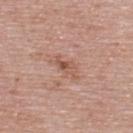<lesion>
  <biopsy_status>not biopsied; imaged during a skin examination</biopsy_status>
  <image>
    <source>total-body photography crop</source>
    <field_of_view_mm>15</field_of_view_mm>
  </image>
  <automated_metrics>
    <area_mm2_approx>4.5</area_mm2_approx>
    <shape_asymmetry>0.4</shape_asymmetry>
  </automated_metrics>
  <patient>
    <sex>female</sex>
    <age_approx>50</age_approx>
  </patient>
  <site>upper back</site>
  <lighting>white-light</lighting>
  <lesion_size>
    <long_diameter_mm_approx>3.0</long_diameter_mm_approx>
  </lesion_size>
</lesion>